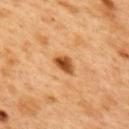The lesion was photographed on a routine skin check and not biopsied; there is no pathology result.
A region of skin cropped from a whole-body photographic capture, roughly 15 mm wide.
Measured at roughly 3 mm in maximum diameter.
On the mid back.
Captured under cross-polarized illumination.
A male subject in their 50s.